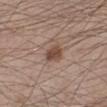The lesion was photographed on a routine skin check and not biopsied; there is no pathology result.
The recorded lesion diameter is about 3 mm.
The subject is a male in their mid- to late 30s.
A region of skin cropped from a whole-body photographic capture, roughly 15 mm wide.
Imaged with white-light lighting.
From the leg.